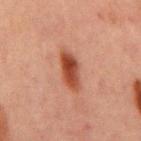| feature | finding |
|---|---|
| follow-up | catalogued during a skin exam; not biopsied |
| TBP lesion metrics | a border-irregularity rating of about 2.5/10 and a within-lesion color-variation index near 5/10 |
| lesion size | ~5 mm (longest diameter) |
| patient | male, approximately 65 years of age |
| anatomic site | the abdomen |
| lighting | cross-polarized |
| image source | total-body-photography crop, ~15 mm field of view |